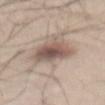No biopsy was performed on this lesion — it was imaged during a full skin examination and was not determined to be concerning. A 15 mm close-up tile from a total-body photography series done for melanoma screening. The lesion is located on the abdomen. This is a white-light tile. An algorithmic analysis of the crop reported a footprint of about 14 mm², an eccentricity of roughly 0.6, and a symmetry-axis asymmetry near 0.25. And it measured an average lesion color of about L≈54 a*≈15 b*≈23 (CIELAB), roughly 13 lightness units darker than nearby skin, and a normalized lesion–skin contrast near 8.5. The recorded lesion diameter is about 4.5 mm. A male subject aged 43–47.